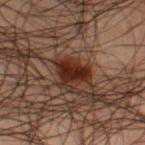Q: Is there a histopathology result?
A: no biopsy performed (imaged during a skin exam)
Q: Illumination type?
A: cross-polarized illumination
Q: What kind of image is this?
A: 15 mm crop, total-body photography
Q: What is the anatomic site?
A: the right lower leg
Q: Patient demographics?
A: male, aged approximately 50
Q: Automated lesion metrics?
A: an area of roughly 8 mm², a shape eccentricity near 0.45, and two-axis asymmetry of about 0.35
Q: How large is the lesion?
A: ≈3.5 mm